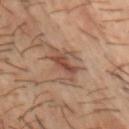Part of a total-body skin-imaging series; this lesion was reviewed on a skin check and was not flagged for biopsy. Located on the chest. A male patient in their 50s. The lesion's longest dimension is about 5 mm. The lesion-visualizer software estimated a lesion area of about 11 mm², a shape eccentricity near 0.8, and a shape-asymmetry score of about 0.45 (0 = symmetric). The analysis additionally found a detector confidence of about 95 out of 100 that the crop contains a lesion. A lesion tile, about 15 mm wide, cut from a 3D total-body photograph.